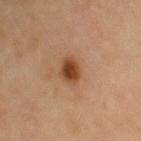Q: Was this lesion biopsied?
A: no biopsy performed (imaged during a skin exam)
Q: How was this image acquired?
A: ~15 mm tile from a whole-body skin photo
Q: What are the patient's age and sex?
A: female, aged approximately 70
Q: Lesion location?
A: the right upper arm
Q: What is the lesion's diameter?
A: ≈3 mm
Q: How was the tile lit?
A: cross-polarized